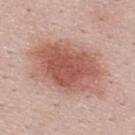This lesion was catalogued during total-body skin photography and was not selected for biopsy.
An algorithmic analysis of the crop reported an area of roughly 43 mm², an eccentricity of roughly 0.75, and a shape-asymmetry score of about 0.2 (0 = symmetric). The software also gave a nevus-likeness score of about 100/100 and a detector confidence of about 100 out of 100 that the crop contains a lesion.
The tile uses white-light illumination.
A male patient in their mid-20s.
From the upper back.
A lesion tile, about 15 mm wide, cut from a 3D total-body photograph.
About 9.5 mm across.